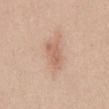| field | value |
|---|---|
| notes | imaged on a skin check; not biopsied |
| subject | female, in their mid- to late 40s |
| automated metrics | about 8 CIELAB-L* units darker than the surrounding skin and a normalized border contrast of about 5.5 |
| body site | the abdomen |
| imaging modality | 15 mm crop, total-body photography |
| size | about 3.5 mm |
| illumination | white-light illumination |